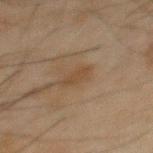Recorded during total-body skin imaging; not selected for excision or biopsy. Automated image analysis of the tile measured an average lesion color of about L≈37 a*≈13 b*≈26 (CIELAB), a lesion–skin lightness drop of about 5, and a lesion-to-skin contrast of about 5.5 (normalized; higher = more distinct). The software also gave an automated nevus-likeness rating near 0 out of 100 and lesion-presence confidence of about 100/100. From the mid back. The subject is a male approximately 45 years of age. Captured under cross-polarized illumination. The recorded lesion diameter is about 3 mm. A lesion tile, about 15 mm wide, cut from a 3D total-body photograph.